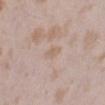Assessment:
Part of a total-body skin-imaging series; this lesion was reviewed on a skin check and was not flagged for biopsy.
Context:
Located on the left lower leg. The lesion's longest dimension is about 2.5 mm. Imaged with white-light lighting. A 15 mm crop from a total-body photograph taken for skin-cancer surveillance. The subject is a female roughly 25 years of age.